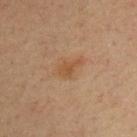Q: Is there a histopathology result?
A: total-body-photography surveillance lesion; no biopsy
Q: What did automated image analysis measure?
A: an area of roughly 3 mm², a shape eccentricity near 0.85, and a symmetry-axis asymmetry near 0.4; a lesion color around L≈46 a*≈19 b*≈33 in CIELAB, roughly 7 lightness units darker than nearby skin, and a normalized lesion–skin contrast near 6.5; border irregularity of about 4 on a 0–10 scale and radial color variation of about 0.5
Q: Lesion location?
A: the chest
Q: What are the patient's age and sex?
A: male, aged 33–37
Q: How was the tile lit?
A: cross-polarized
Q: How large is the lesion?
A: ~2.5 mm (longest diameter)
Q: What is the imaging modality?
A: ~15 mm crop, total-body skin-cancer survey